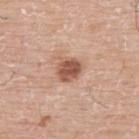biopsy status = no biopsy performed (imaged during a skin exam) | imaging modality = 15 mm crop, total-body photography | location = the upper back | lighting = white-light illumination | lesion size = ~3 mm (longest diameter) | automated lesion analysis = a lesion area of about 7 mm², a shape eccentricity near 0.5, and two-axis asymmetry of about 0.2; a lesion color around L≈55 a*≈22 b*≈29 in CIELAB, roughly 14 lightness units darker than nearby skin, and a normalized lesion–skin contrast near 9; a border-irregularity rating of about 2/10, a within-lesion color-variation index near 3.5/10, and radial color variation of about 1.5; an automated nevus-likeness rating near 85 out of 100 and a detector confidence of about 100 out of 100 that the crop contains a lesion | patient = male, in their mid- to late 70s.